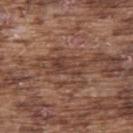biopsy_status: not biopsied; imaged during a skin examination
lighting: white-light
automated_metrics:
  area_mm2_approx: 6.0
  eccentricity: 0.9
  shape_asymmetry: 0.45
  border_irregularity_0_10: 5.5
  color_variation_0_10: 3.0
  peripheral_color_asymmetry: 1.0
  lesion_detection_confidence_0_100: 65
site: upper back
patient:
  sex: male
  age_approx: 75
lesion_size:
  long_diameter_mm_approx: 4.0
image:
  source: total-body photography crop
  field_of_view_mm: 15